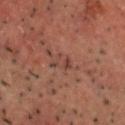Notes:
* illumination · cross-polarized illumination
* subject · male, roughly 50 years of age
* acquisition · 15 mm crop, total-body photography
* lesion diameter · ~3 mm (longest diameter)
* anatomic site · the head or neck